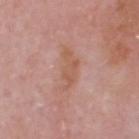Impression:
The lesion was photographed on a routine skin check and not biopsied; there is no pathology result.
Background:
An algorithmic analysis of the crop reported a footprint of about 5.5 mm² and an eccentricity of roughly 0.85. The analysis additionally found a lesion color around L≈56 a*≈22 b*≈30 in CIELAB and a lesion–skin lightness drop of about 7. The software also gave a border-irregularity index near 6.5/10, a color-variation rating of about 1/10, and peripheral color asymmetry of about 0. A 15 mm crop from a total-body photograph taken for skin-cancer surveillance. This is a white-light tile. Located on the head or neck. Longest diameter approximately 4 mm. A male patient in their 60s.